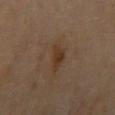Q: Was this lesion biopsied?
A: imaged on a skin check; not biopsied
Q: How was this image acquired?
A: ~15 mm crop, total-body skin-cancer survey
Q: Lesion location?
A: the right upper arm
Q: Lesion size?
A: about 3 mm
Q: Patient demographics?
A: male, aged around 85 or older